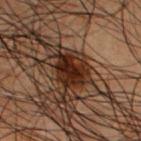Assessment: Imaged during a routine full-body skin examination; the lesion was not biopsied and no histopathology is available. Background: This is a cross-polarized tile. Measured at roughly 5.5 mm in maximum diameter. A 15 mm close-up extracted from a 3D total-body photography capture. The lesion-visualizer software estimated an area of roughly 17 mm², an outline eccentricity of about 0.6 (0 = round, 1 = elongated), and two-axis asymmetry of about 0.3. It also reported a lesion color around L≈20 a*≈15 b*≈20 in CIELAB and roughly 10 lightness units darker than nearby skin. The software also gave a border-irregularity rating of about 4/10 and internal color variation of about 6 on a 0–10 scale. A male patient aged around 50. Located on the chest.